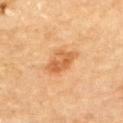{
  "biopsy_status": "not biopsied; imaged during a skin examination",
  "patient": {
    "sex": "male",
    "age_approx": "85+"
  },
  "image": {
    "source": "total-body photography crop",
    "field_of_view_mm": 15
  },
  "lesion_size": {
    "long_diameter_mm_approx": 4.0
  },
  "automated_metrics": {
    "area_mm2_approx": 8.0,
    "eccentricity": 0.75,
    "shape_asymmetry": 0.25
  },
  "site": "upper back",
  "lighting": "cross-polarized"
}